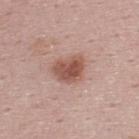| feature | finding |
|---|---|
| size | about 3.5 mm |
| image | ~15 mm crop, total-body skin-cancer survey |
| subject | male, aged 23 to 27 |
| body site | the upper back |